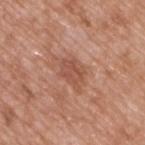This lesion was catalogued during total-body skin photography and was not selected for biopsy.
Automated image analysis of the tile measured a mean CIELAB color near L≈52 a*≈24 b*≈29, roughly 8 lightness units darker than nearby skin, and a normalized border contrast of about 6. The analysis additionally found a classifier nevus-likeness of about 0/100.
This image is a 15 mm lesion crop taken from a total-body photograph.
The lesion is on the back.
A male patient, aged 48–52.